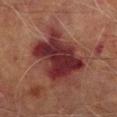The lesion was photographed on a routine skin check and not biopsied; there is no pathology result. The recorded lesion diameter is about 8 mm. This image is a 15 mm lesion crop taken from a total-body photograph. On the right lower leg. A male patient about 70 years old. Imaged with cross-polarized lighting.